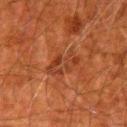Measured at roughly 5 mm in maximum diameter.
The subject is a male aged around 80.
The lesion is on the arm.
The tile uses cross-polarized illumination.
The lesion-visualizer software estimated border irregularity of about 4.5 on a 0–10 scale, internal color variation of about 4.5 on a 0–10 scale, and radial color variation of about 1.5. The software also gave a nevus-likeness score of about 0/100 and lesion-presence confidence of about 90/100.
A 15 mm close-up extracted from a 3D total-body photography capture.